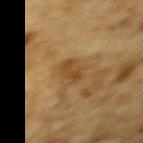Notes:
- workup: no biopsy performed (imaged during a skin exam)
- imaging modality: ~15 mm crop, total-body skin-cancer survey
- lighting: cross-polarized illumination
- patient: male, in their mid-80s or older
- site: the upper back